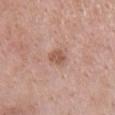Q: Is there a histopathology result?
A: catalogued during a skin exam; not biopsied
Q: What kind of image is this?
A: ~15 mm crop, total-body skin-cancer survey
Q: Lesion size?
A: ~2.5 mm (longest diameter)
Q: Automated lesion metrics?
A: a mean CIELAB color near L≈56 a*≈21 b*≈28 and a normalized border contrast of about 7; a border-irregularity index near 2.5/10, a color-variation rating of about 3/10, and radial color variation of about 1
Q: Illumination type?
A: white-light
Q: What are the patient's age and sex?
A: female, aged 43 to 47
Q: Lesion location?
A: the chest